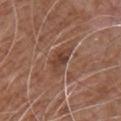Q: Was this lesion biopsied?
A: imaged on a skin check; not biopsied
Q: How was this image acquired?
A: total-body-photography crop, ~15 mm field of view
Q: Lesion location?
A: the upper back
Q: Lesion size?
A: ~4 mm (longest diameter)
Q: Who is the patient?
A: male, roughly 60 years of age
Q: Automated lesion metrics?
A: a footprint of about 6 mm², an eccentricity of roughly 0.85, and a symmetry-axis asymmetry near 0.3; a mean CIELAB color near L≈42 a*≈21 b*≈27, about 9 CIELAB-L* units darker than the surrounding skin, and a lesion-to-skin contrast of about 7 (normalized; higher = more distinct); a border-irregularity index near 4/10, internal color variation of about 3.5 on a 0–10 scale, and peripheral color asymmetry of about 1; a nevus-likeness score of about 0/100 and a lesion-detection confidence of about 95/100